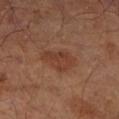Impression: No biopsy was performed on this lesion — it was imaged during a full skin examination and was not determined to be concerning. Image and clinical context: Measured at roughly 4 mm in maximum diameter. This is a cross-polarized tile. Located on the right lower leg. The patient is a male aged approximately 65. A 15 mm crop from a total-body photograph taken for skin-cancer surveillance. The total-body-photography lesion software estimated a lesion area of about 9 mm², an outline eccentricity of about 0.65 (0 = round, 1 = elongated), and a symmetry-axis asymmetry near 0.35. The analysis additionally found about 7 CIELAB-L* units darker than the surrounding skin. The software also gave border irregularity of about 3.5 on a 0–10 scale, internal color variation of about 2.5 on a 0–10 scale, and a peripheral color-asymmetry measure near 1. And it measured a classifier nevus-likeness of about 60/100 and a lesion-detection confidence of about 100/100.